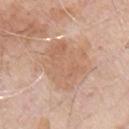Case summary:
• follow-up: no biopsy performed (imaged during a skin exam)
• size: about 5.5 mm
• image-analysis metrics: an area of roughly 21 mm², a shape eccentricity near 0.35, and two-axis asymmetry of about 0.2; an average lesion color of about L≈62 a*≈19 b*≈31 (CIELAB), a lesion–skin lightness drop of about 7, and a normalized border contrast of about 5.5; a nevus-likeness score of about 0/100 and lesion-presence confidence of about 100/100
• illumination: white-light
• acquisition: total-body-photography crop, ~15 mm field of view
• subject: male, aged around 70
• anatomic site: the chest